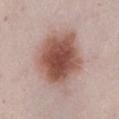Imaged during a routine full-body skin examination; the lesion was not biopsied and no histopathology is available.